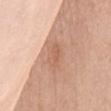Captured during whole-body skin photography for melanoma surveillance; the lesion was not biopsied. A close-up tile cropped from a whole-body skin photograph, about 15 mm across. On the chest. Automated tile analysis of the lesion measured a mean CIELAB color near L≈60 a*≈22 b*≈32, roughly 8 lightness units darker than nearby skin, and a normalized lesion–skin contrast near 5.5. And it measured a border-irregularity index near 3.5/10, a color-variation rating of about 1.5/10, and a peripheral color-asymmetry measure near 0.5. The software also gave a classifier nevus-likeness of about 0/100 and a detector confidence of about 95 out of 100 that the crop contains a lesion. The patient is a female approximately 45 years of age. Approximately 3.5 mm at its widest.